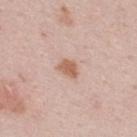{"biopsy_status": "not biopsied; imaged during a skin examination", "image": {"source": "total-body photography crop", "field_of_view_mm": 15}, "site": "upper back", "patient": {"sex": "male", "age_approx": 25}}